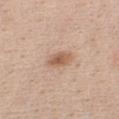body site: the chest | subject: female, aged around 40 | lesion size: ≈3.5 mm | image source: ~15 mm crop, total-body skin-cancer survey.